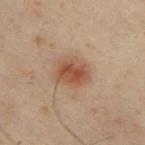<record>
<biopsy_status>not biopsied; imaged during a skin examination</biopsy_status>
<image>
  <source>total-body photography crop</source>
  <field_of_view_mm>15</field_of_view_mm>
</image>
<lesion_size>
  <long_diameter_mm_approx>4.0</long_diameter_mm_approx>
</lesion_size>
<site>upper back</site>
<lighting>cross-polarized</lighting>
<patient>
  <sex>male</sex>
  <age_approx>50</age_approx>
</patient>
</record>